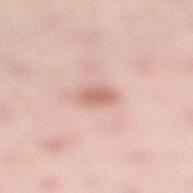Clinical impression: The lesion was tiled from a total-body skin photograph and was not biopsied. Context: Measured at roughly 3 mm in maximum diameter. The subject is a female aged 28 to 32. Captured under white-light illumination. The lesion is on the leg. A lesion tile, about 15 mm wide, cut from a 3D total-body photograph. The total-body-photography lesion software estimated a lesion area of about 4 mm². The software also gave an average lesion color of about L≈65 a*≈22 b*≈28 (CIELAB), a lesion–skin lightness drop of about 11, and a lesion-to-skin contrast of about 7 (normalized; higher = more distinct). The software also gave border irregularity of about 2 on a 0–10 scale and a peripheral color-asymmetry measure near 0.5.